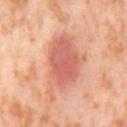Clinical impression: Part of a total-body skin-imaging series; this lesion was reviewed on a skin check and was not flagged for biopsy.